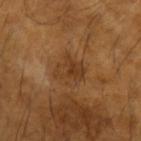<case>
  <biopsy_status>not biopsied; imaged during a skin examination</biopsy_status>
  <image>
    <source>total-body photography crop</source>
    <field_of_view_mm>15</field_of_view_mm>
  </image>
  <patient>
    <sex>male</sex>
    <age_approx>65</age_approx>
  </patient>
  <site>left forearm</site>
</case>